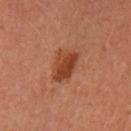<case>
  <biopsy_status>not biopsied; imaged during a skin examination</biopsy_status>
</case>